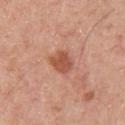lesion diameter: ~3 mm (longest diameter) | patient: male, aged 58 to 62 | anatomic site: the chest | TBP lesion metrics: an area of roughly 6 mm², an eccentricity of roughly 0.5, and a symmetry-axis asymmetry near 0.2; an average lesion color of about L≈53 a*≈27 b*≈32 (CIELAB), about 11 CIELAB-L* units darker than the surrounding skin, and a lesion-to-skin contrast of about 8 (normalized; higher = more distinct) | imaging modality: ~15 mm crop, total-body skin-cancer survey.